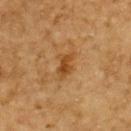Q: Where on the body is the lesion?
A: the upper back
Q: Patient demographics?
A: male, in their mid- to late 80s or older
Q: Illumination type?
A: cross-polarized
Q: What kind of image is this?
A: ~15 mm crop, total-body skin-cancer survey
Q: Automated lesion metrics?
A: a mean CIELAB color near L≈40 a*≈20 b*≈37, a lesion–skin lightness drop of about 8, and a normalized border contrast of about 7.5; a border-irregularity rating of about 4.5/10